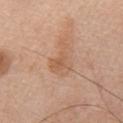notes = total-body-photography surveillance lesion; no biopsy | illumination = white-light | patient = male, in their mid-70s | imaging modality = 15 mm crop, total-body photography | body site = the chest.